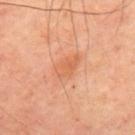{"biopsy_status": "not biopsied; imaged during a skin examination", "image": {"source": "total-body photography crop", "field_of_view_mm": 15}, "site": "back", "patient": {"sex": "male", "age_approx": 50}, "lighting": "cross-polarized"}